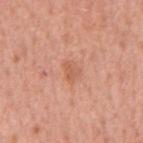workup: total-body-photography surveillance lesion; no biopsy
patient: male, roughly 65 years of age
TBP lesion metrics: border irregularity of about 3.5 on a 0–10 scale, a color-variation rating of about 1.5/10, and a peripheral color-asymmetry measure near 0.5; a classifier nevus-likeness of about 0/100 and lesion-presence confidence of about 100/100
imaging modality: 15 mm crop, total-body photography
lesion diameter: ≈2.5 mm
anatomic site: the mid back